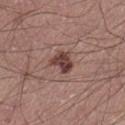Q: Was a biopsy performed?
A: imaged on a skin check; not biopsied
Q: Where on the body is the lesion?
A: the left thigh
Q: What is the lesion's diameter?
A: ≈3 mm
Q: What are the patient's age and sex?
A: male, roughly 25 years of age
Q: What is the imaging modality?
A: total-body-photography crop, ~15 mm field of view
Q: What lighting was used for the tile?
A: white-light illumination